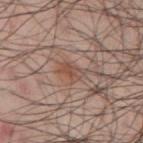lighting: white-light illumination
imaging modality: ~15 mm tile from a whole-body skin photo
location: the right upper arm
subject: male, aged approximately 45
diameter: ≈3 mm